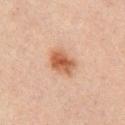biopsy status = catalogued during a skin exam; not biopsied
subject = male, aged 28 to 32
body site = the chest
imaging modality = 15 mm crop, total-body photography